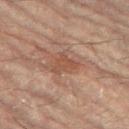Assessment: This lesion was catalogued during total-body skin photography and was not selected for biopsy. Clinical summary: A lesion tile, about 15 mm wide, cut from a 3D total-body photograph. From the right leg. About 3 mm across. This is a cross-polarized tile. A female patient, aged around 80. Automated image analysis of the tile measured a border-irregularity rating of about 4.5/10, a within-lesion color-variation index near 1.5/10, and peripheral color asymmetry of about 0.5.